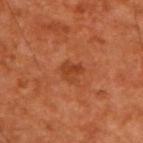follow-up — total-body-photography surveillance lesion; no biopsy
lesion diameter — ≈2.5 mm
site — the upper back
lighting — cross-polarized illumination
image — 15 mm crop, total-body photography
subject — male, aged around 65